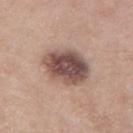Clinical impression: This lesion was catalogued during total-body skin photography and was not selected for biopsy. Acquisition and patient details: Cropped from a whole-body photographic skin survey; the tile spans about 15 mm. The lesion is located on the left thigh. About 5.5 mm across. An algorithmic analysis of the crop reported a mean CIELAB color near L≈50 a*≈18 b*≈22, roughly 16 lightness units darker than nearby skin, and a normalized border contrast of about 11.5. And it measured a nevus-likeness score of about 25/100. The tile uses white-light illumination. The subject is a female aged 53–57.